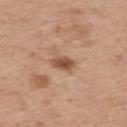Impression:
The lesion was tiled from a total-body skin photograph and was not biopsied.
Clinical summary:
A region of skin cropped from a whole-body photographic capture, roughly 15 mm wide. From the upper back. A male patient, in their mid- to late 70s.